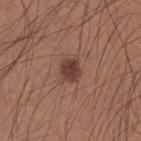Case summary:
- follow-up — catalogued during a skin exam; not biopsied
- body site — the mid back
- patient — male, roughly 35 years of age
- image source — 15 mm crop, total-body photography
- size — ~2.5 mm (longest diameter)